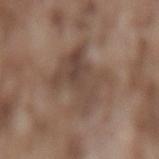The lesion was tiled from a total-body skin photograph and was not biopsied. The lesion is on the lower back. A male subject, aged around 75. This image is a 15 mm lesion crop taken from a total-body photograph.